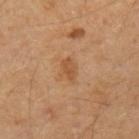Image and clinical context: This is a cross-polarized tile. Approximately 2.5 mm at its widest. A male patient, aged approximately 40. Automated image analysis of the tile measured a shape eccentricity near 0.85 and two-axis asymmetry of about 0.2. The analysis additionally found a lesion color around L≈52 a*≈22 b*≈37 in CIELAB and a normalized lesion–skin contrast near 6.5. And it measured a nevus-likeness score of about 30/100 and a lesion-detection confidence of about 100/100. From the left upper arm. A lesion tile, about 15 mm wide, cut from a 3D total-body photograph.